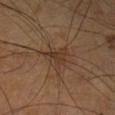Assessment:
This lesion was catalogued during total-body skin photography and was not selected for biopsy.
Clinical summary:
The lesion is located on the left lower leg. Cropped from a whole-body photographic skin survey; the tile spans about 15 mm. This is a cross-polarized tile. The recorded lesion diameter is about 4 mm. A male subject aged around 65.